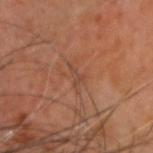{
  "biopsy_status": "not biopsied; imaged during a skin examination",
  "lesion_size": {
    "long_diameter_mm_approx": 3.0
  },
  "image": {
    "source": "total-body photography crop",
    "field_of_view_mm": 15
  },
  "patient": {
    "sex": "male",
    "age_approx": 60
  },
  "lighting": "cross-polarized",
  "site": "head or neck"
}